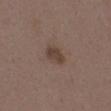Image and clinical context: Automated image analysis of the tile measured a border-irregularity index near 2.5/10 and a peripheral color-asymmetry measure near 1. It also reported a classifier nevus-likeness of about 40/100 and lesion-presence confidence of about 100/100. The lesion is on the chest. This is a white-light tile. A female patient, roughly 35 years of age. The recorded lesion diameter is about 3 mm. This image is a 15 mm lesion crop taken from a total-body photograph.